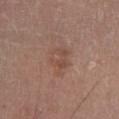biopsy_status: not biopsied; imaged during a skin examination
site: left lower leg
automated_metrics:
  area_mm2_approx: 8.0
  eccentricity: 0.65
  shape_asymmetry: 0.3
  border_irregularity_0_10: 3.5
  color_variation_0_10: 4.0
  peripheral_color_asymmetry: 1.5
lighting: white-light
lesion_size:
  long_diameter_mm_approx: 3.5
image:
  source: total-body photography crop
  field_of_view_mm: 15
patient:
  sex: male
  age_approx: 55